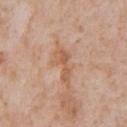Assessment: No biopsy was performed on this lesion — it was imaged during a full skin examination and was not determined to be concerning.